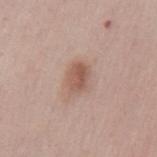- biopsy status — no biopsy performed (imaged during a skin exam)
- imaging modality — total-body-photography crop, ~15 mm field of view
- site — the mid back
- patient — male, in their mid-70s